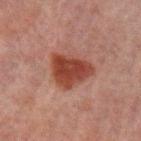notes = catalogued during a skin exam; not biopsied
image source = ~15 mm crop, total-body skin-cancer survey
anatomic site = the arm
subject = male, in their 60s
illumination = cross-polarized